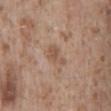biopsy_status: not biopsied; imaged during a skin examination
image:
  source: total-body photography crop
  field_of_view_mm: 15
lighting: white-light
lesion_size:
  long_diameter_mm_approx: 3.0
site: mid back
patient:
  sex: male
  age_approx: 45